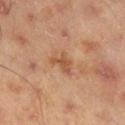<record>
  <biopsy_status>not biopsied; imaged during a skin examination</biopsy_status>
  <site>right lower leg</site>
  <image>
    <source>total-body photography crop</source>
    <field_of_view_mm>15</field_of_view_mm>
  </image>
  <lighting>cross-polarized</lighting>
  <lesion_size>
    <long_diameter_mm_approx>3.0</long_diameter_mm_approx>
  </lesion_size>
  <patient>
    <sex>male</sex>
    <age_approx>45</age_approx>
  </patient>
  <automated_metrics>
    <cielab_L>53</cielab_L>
    <cielab_a>22</cielab_a>
    <cielab_b>35</cielab_b>
    <vs_skin_darker_L>9.0</vs_skin_darker_L>
    <vs_skin_contrast_norm>6.5</vs_skin_contrast_norm>
    <border_irregularity_0_10>5.0</border_irregularity_0_10>
    <color_variation_0_10>1.5</color_variation_0_10>
    <peripheral_color_asymmetry>0.5</peripheral_color_asymmetry>
    <nevus_likeness_0_100>0</nevus_likeness_0_100>
    <lesion_detection_confidence_0_100>100</lesion_detection_confidence_0_100>
  </automated_metrics>
</record>